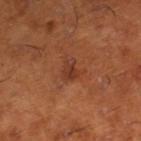<record>
<biopsy_status>not biopsied; imaged during a skin examination</biopsy_status>
<image>
  <source>total-body photography crop</source>
  <field_of_view_mm>15</field_of_view_mm>
</image>
<lighting>cross-polarized</lighting>
<lesion_size>
  <long_diameter_mm_approx>2.5</long_diameter_mm_approx>
</lesion_size>
<site>right lower leg</site>
<automated_metrics>
  <area_mm2_approx>4.0</area_mm2_approx>
  <shape_asymmetry>0.35</shape_asymmetry>
  <cielab_L>37</cielab_L>
  <cielab_a>26</cielab_a>
  <cielab_b>32</cielab_b>
  <vs_skin_darker_L>7.0</vs_skin_darker_L>
  <vs_skin_contrast_norm>6.5</vs_skin_contrast_norm>
  <border_irregularity_0_10>3.0</border_irregularity_0_10>
  <color_variation_0_10>2.5</color_variation_0_10>
  <peripheral_color_asymmetry>1.0</peripheral_color_asymmetry>
</automated_metrics>
<patient>
  <sex>male</sex>
  <age_approx>65</age_approx>
</patient>
</record>